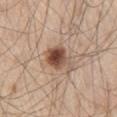The lesion was photographed on a routine skin check and not biopsied; there is no pathology result.
A male subject aged 58 to 62.
A lesion tile, about 15 mm wide, cut from a 3D total-body photograph.
On the leg.
Approximately 4 mm at its widest.
Captured under white-light illumination.